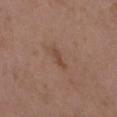<record>
  <biopsy_status>not biopsied; imaged during a skin examination</biopsy_status>
  <image>
    <source>total-body photography crop</source>
    <field_of_view_mm>15</field_of_view_mm>
  </image>
  <site>arm</site>
  <lighting>white-light</lighting>
  <patient>
    <sex>female</sex>
    <age_approx>30</age_approx>
  </patient>
  <lesion_size>
    <long_diameter_mm_approx>2.5</long_diameter_mm_approx>
  </lesion_size>
</record>